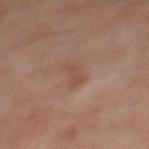Imaged during a routine full-body skin examination; the lesion was not biopsied and no histopathology is available.
A 15 mm crop from a total-body photograph taken for skin-cancer surveillance.
A male subject, aged 48 to 52.
From the back.
The lesion's longest dimension is about 3 mm.